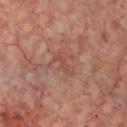Impression:
Captured during whole-body skin photography for melanoma surveillance; the lesion was not biopsied.
Image and clinical context:
An algorithmic analysis of the crop reported an average lesion color of about L≈47 a*≈23 b*≈28 (CIELAB), a lesion–skin lightness drop of about 6, and a normalized border contrast of about 5. And it measured border irregularity of about 4.5 on a 0–10 scale, a color-variation rating of about 0/10, and peripheral color asymmetry of about 0. A close-up tile cropped from a whole-body skin photograph, about 15 mm across. Longest diameter approximately 3.5 mm. The patient is in their mid-60s. The lesion is located on the front of the torso.